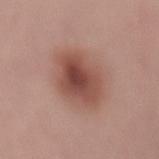Clinical impression:
Recorded during total-body skin imaging; not selected for excision or biopsy.
Context:
The patient is a male aged around 30. From the lower back. A 15 mm crop from a total-body photograph taken for skin-cancer surveillance.